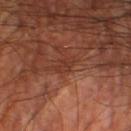notes — catalogued during a skin exam; not biopsied
lighting — cross-polarized
subject — male, approximately 60 years of age
location — the right lower leg
lesion diameter — ~2.5 mm (longest diameter)
image source — 15 mm crop, total-body photography